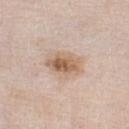{
  "biopsy_status": "not biopsied; imaged during a skin examination",
  "patient": {
    "sex": "male",
    "age_approx": 80
  },
  "lighting": "white-light",
  "automated_metrics": {
    "cielab_L": 62,
    "cielab_a": 17,
    "cielab_b": 30,
    "vs_skin_darker_L": 11.0,
    "vs_skin_contrast_norm": 8.0,
    "border_irregularity_0_10": 2.0,
    "color_variation_0_10": 6.5,
    "peripheral_color_asymmetry": 2.0
  },
  "site": "left lower leg",
  "lesion_size": {
    "long_diameter_mm_approx": 4.5
  },
  "image": {
    "source": "total-body photography crop",
    "field_of_view_mm": 15
  }
}